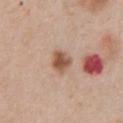{
  "biopsy_status": "not biopsied; imaged during a skin examination",
  "site": "front of the torso",
  "image": {
    "source": "total-body photography crop",
    "field_of_view_mm": 15
  },
  "automated_metrics": {
    "border_irregularity_0_10": 2.0,
    "color_variation_0_10": 4.0,
    "nevus_likeness_0_100": 95
  },
  "patient": {
    "sex": "male",
    "age_approx": 60
  },
  "lesion_size": {
    "long_diameter_mm_approx": 2.5
  },
  "lighting": "white-light"
}